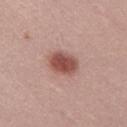follow-up = no biopsy performed (imaged during a skin exam); subject = male, aged 28 to 32; lesion size = ~4 mm (longest diameter); image = ~15 mm crop, total-body skin-cancer survey; lighting = white-light illumination.